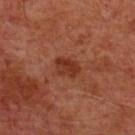Q: Who is the patient?
A: male, aged 58–62
Q: What lighting was used for the tile?
A: cross-polarized
Q: What kind of image is this?
A: ~15 mm tile from a whole-body skin photo
Q: What is the anatomic site?
A: the chest
Q: What is the lesion's diameter?
A: about 3 mm